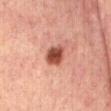The lesion was tiled from a total-body skin photograph and was not biopsied.
From the abdomen.
This is a cross-polarized tile.
Cropped from a whole-body photographic skin survey; the tile spans about 15 mm.
The subject is a male in their mid-60s.